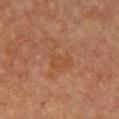Clinical impression:
Recorded during total-body skin imaging; not selected for excision or biopsy.
Acquisition and patient details:
The tile uses cross-polarized illumination. The lesion-visualizer software estimated a footprint of about 7 mm², an outline eccentricity of about 0.8 (0 = round, 1 = elongated), and a symmetry-axis asymmetry near 0.5. The analysis additionally found an average lesion color of about L≈40 a*≈20 b*≈30 (CIELAB), about 4 CIELAB-L* units darker than the surrounding skin, and a normalized lesion–skin contrast near 4.5. And it measured border irregularity of about 7 on a 0–10 scale and internal color variation of about 2 on a 0–10 scale. Longest diameter approximately 4.5 mm. On the front of the torso. A 15 mm close-up tile from a total-body photography series done for melanoma screening. A female patient aged approximately 70.